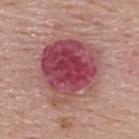| feature | finding |
|---|---|
| site | the back |
| illumination | white-light illumination |
| image source | total-body-photography crop, ~15 mm field of view |
| lesion diameter | ~7.5 mm (longest diameter) |
| patient | male, roughly 55 years of age |